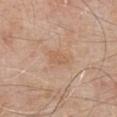The recorded lesion diameter is about 2.5 mm. The subject is a male aged 78–82. Cropped from a whole-body photographic skin survey; the tile spans about 15 mm. The tile uses white-light illumination. On the chest.